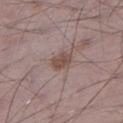<case>
  <biopsy_status>not biopsied; imaged during a skin examination</biopsy_status>
  <lighting>white-light</lighting>
  <lesion_size>
    <long_diameter_mm_approx>3.0</long_diameter_mm_approx>
  </lesion_size>
  <automated_metrics>
    <area_mm2_approx>5.0</area_mm2_approx>
    <shape_asymmetry>0.2</shape_asymmetry>
    <nevus_likeness_0_100>40</nevus_likeness_0_100>
  </automated_metrics>
  <site>left lower leg</site>
  <image>
    <source>total-body photography crop</source>
    <field_of_view_mm>15</field_of_view_mm>
  </image>
  <patient>
    <sex>male</sex>
    <age_approx>70</age_approx>
  </patient>
</case>